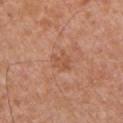{
  "biopsy_status": "not biopsied; imaged during a skin examination",
  "image": {
    "source": "total-body photography crop",
    "field_of_view_mm": 15
  },
  "site": "chest",
  "lighting": "white-light",
  "patient": {
    "sex": "male",
    "age_approx": 30
  },
  "automated_metrics": {
    "area_mm2_approx": 5.5,
    "vs_skin_darker_L": 6.0,
    "vs_skin_contrast_norm": 5.0,
    "border_irregularity_0_10": 3.5,
    "color_variation_0_10": 2.0,
    "peripheral_color_asymmetry": 1.0
  },
  "lesion_size": {
    "long_diameter_mm_approx": 3.0
  }
}